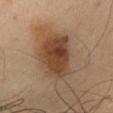Part of a total-body skin-imaging series; this lesion was reviewed on a skin check and was not flagged for biopsy.
From the chest.
Measured at roughly 7 mm in maximum diameter.
An algorithmic analysis of the crop reported an average lesion color of about L≈41 a*≈18 b*≈30 (CIELAB), about 13 CIELAB-L* units darker than the surrounding skin, and a lesion-to-skin contrast of about 10.5 (normalized; higher = more distinct). The analysis additionally found a border-irregularity rating of about 2.5/10 and a color-variation rating of about 5/10. The software also gave an automated nevus-likeness rating near 100 out of 100 and a detector confidence of about 100 out of 100 that the crop contains a lesion.
A male patient, roughly 60 years of age.
A 15 mm crop from a total-body photograph taken for skin-cancer surveillance.
Imaged with cross-polarized lighting.